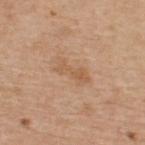{
  "biopsy_status": "not biopsied; imaged during a skin examination",
  "lighting": "white-light",
  "automated_metrics": {
    "cielab_L": 58,
    "cielab_a": 19,
    "cielab_b": 34,
    "vs_skin_darker_L": 7.0,
    "border_irregularity_0_10": 4.5,
    "peripheral_color_asymmetry": 0.5,
    "nevus_likeness_0_100": 0,
    "lesion_detection_confidence_0_100": 100
  },
  "patient": {
    "sex": "male",
    "age_approx": 65
  },
  "image": {
    "source": "total-body photography crop",
    "field_of_view_mm": 15
  },
  "site": "upper back",
  "lesion_size": {
    "long_diameter_mm_approx": 4.0
  }
}